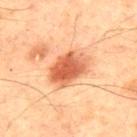Q: Was this lesion biopsied?
A: imaged on a skin check; not biopsied
Q: How was the tile lit?
A: cross-polarized
Q: Automated lesion metrics?
A: an outline eccentricity of about 0.75 (0 = round, 1 = elongated) and two-axis asymmetry of about 0.15; an average lesion color of about L≈50 a*≈25 b*≈33 (CIELAB), about 13 CIELAB-L* units darker than the surrounding skin, and a normalized border contrast of about 9; a border-irregularity rating of about 2/10 and internal color variation of about 5.5 on a 0–10 scale
Q: Lesion location?
A: the front of the torso
Q: How was this image acquired?
A: ~15 mm crop, total-body skin-cancer survey
Q: What are the patient's age and sex?
A: male, in their mid- to late 60s
Q: What is the lesion's diameter?
A: ~5 mm (longest diameter)